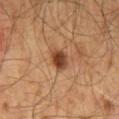Imaged during a routine full-body skin examination; the lesion was not biopsied and no histopathology is available.
An algorithmic analysis of the crop reported a shape eccentricity near 0.65 and two-axis asymmetry of about 0.15. The analysis additionally found a mean CIELAB color near L≈39 a*≈21 b*≈31, a lesion–skin lightness drop of about 14, and a lesion-to-skin contrast of about 11 (normalized; higher = more distinct). The software also gave peripheral color asymmetry of about 1.
The lesion's longest dimension is about 2.5 mm.
A male subject about 60 years old.
A 15 mm close-up tile from a total-body photography series done for melanoma screening.
From the back.
The tile uses cross-polarized illumination.